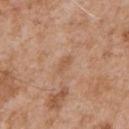Image and clinical context: On the front of the torso. A male subject aged approximately 65. A lesion tile, about 15 mm wide, cut from a 3D total-body photograph. Measured at roughly 2.5 mm in maximum diameter.